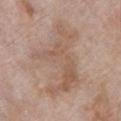Clinical impression:
The lesion was photographed on a routine skin check and not biopsied; there is no pathology result.
Background:
A roughly 15 mm field-of-view crop from a total-body skin photograph. Longest diameter approximately 9.5 mm. A male patient, aged 78 to 82. Located on the chest. Imaged with white-light lighting.